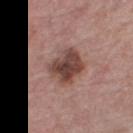Part of a total-body skin-imaging series; this lesion was reviewed on a skin check and was not flagged for biopsy.
This is a white-light tile.
An algorithmic analysis of the crop reported an area of roughly 13 mm², an outline eccentricity of about 0.4 (0 = round, 1 = elongated), and a symmetry-axis asymmetry near 0.15. It also reported a lesion color around L≈44 a*≈20 b*≈23 in CIELAB and a lesion-to-skin contrast of about 10 (normalized; higher = more distinct). The analysis additionally found a classifier nevus-likeness of about 40/100 and a detector confidence of about 100 out of 100 that the crop contains a lesion.
A female patient roughly 65 years of age.
The recorded lesion diameter is about 4 mm.
The lesion is on the right thigh.
A region of skin cropped from a whole-body photographic capture, roughly 15 mm wide.